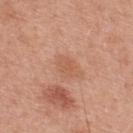Captured during whole-body skin photography for melanoma surveillance; the lesion was not biopsied. A male subject aged around 30. The lesion is on the upper back. The recorded lesion diameter is about 3 mm. Cropped from a whole-body photographic skin survey; the tile spans about 15 mm.